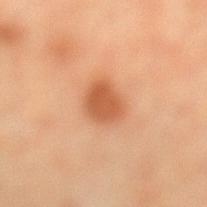- follow-up: total-body-photography surveillance lesion; no biopsy
- image source: ~15 mm tile from a whole-body skin photo
- site: the left lower leg
- illumination: cross-polarized
- patient: female, approximately 55 years of age
- lesion size: about 3.5 mm
- automated metrics: a lesion color around L≈50 a*≈24 b*≈35 in CIELAB, about 11 CIELAB-L* units darker than the surrounding skin, and a normalized border contrast of about 8; a border-irregularity index near 1.5/10 and a color-variation rating of about 2/10; a nevus-likeness score of about 100/100 and lesion-presence confidence of about 100/100